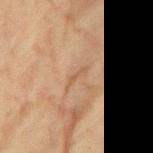Assessment:
No biopsy was performed on this lesion — it was imaged during a full skin examination and was not determined to be concerning.
Image and clinical context:
The subject is a female approximately 80 years of age. This image is a 15 mm lesion crop taken from a total-body photograph. Located on the left forearm.